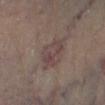workup: total-body-photography surveillance lesion; no biopsy
lighting: cross-polarized illumination
automated lesion analysis: an area of roughly 8.5 mm² and an eccentricity of roughly 0.7; a lesion color around L≈40 a*≈14 b*≈17 in CIELAB, about 6 CIELAB-L* units darker than the surrounding skin, and a lesion-to-skin contrast of about 5.5 (normalized; higher = more distinct)
location: the left lower leg
image source: total-body-photography crop, ~15 mm field of view
subject: male, approximately 85 years of age or older
lesion diameter: ≈4 mm